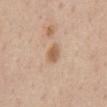{
  "biopsy_status": "not biopsied; imaged during a skin examination",
  "automated_metrics": {
    "border_irregularity_0_10": 2.0,
    "color_variation_0_10": 2.5,
    "peripheral_color_asymmetry": 1.0,
    "nevus_likeness_0_100": 75,
    "lesion_detection_confidence_0_100": 100
  },
  "patient": {
    "sex": "male",
    "age_approx": 60
  },
  "site": "chest",
  "lighting": "white-light",
  "lesion_size": {
    "long_diameter_mm_approx": 2.5
  },
  "image": {
    "source": "total-body photography crop",
    "field_of_view_mm": 15
  }
}